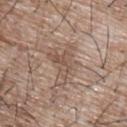{
  "biopsy_status": "not biopsied; imaged during a skin examination",
  "image": {
    "source": "total-body photography crop",
    "field_of_view_mm": 15
  },
  "patient": {
    "sex": "male",
    "age_approx": 65
  },
  "site": "back",
  "lighting": "white-light",
  "lesion_size": {
    "long_diameter_mm_approx": 5.0
  }
}